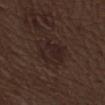workup — total-body-photography surveillance lesion; no biopsy | imaging modality — 15 mm crop, total-body photography | subject — male, roughly 70 years of age | illumination — white-light illumination | lesion diameter — ≈4 mm | location — the right lower leg.